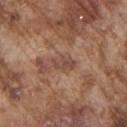The lesion was photographed on a routine skin check and not biopsied; there is no pathology result.
Cropped from a whole-body photographic skin survey; the tile spans about 15 mm.
A male patient about 75 years old.
Longest diameter approximately 3 mm.
The lesion is located on the chest.